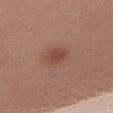Acquisition and patient details:
The patient is a female approximately 25 years of age. An algorithmic analysis of the crop reported a shape-asymmetry score of about 0.1 (0 = symmetric). The analysis additionally found roughly 8 lightness units darker than nearby skin and a normalized border contrast of about 6.5. And it measured border irregularity of about 1 on a 0–10 scale, a color-variation rating of about 2.5/10, and peripheral color asymmetry of about 1. It also reported a classifier nevus-likeness of about 95/100 and lesion-presence confidence of about 100/100. A region of skin cropped from a whole-body photographic capture, roughly 15 mm wide. The recorded lesion diameter is about 3 mm. From the right thigh.